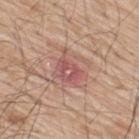  biopsy_status: not biopsied; imaged during a skin examination
  image:
    source: total-body photography crop
    field_of_view_mm: 15
  site: back
  patient:
    sex: male
    age_approx: 70
  lighting: white-light